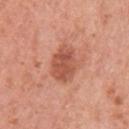Assessment:
Imaged during a routine full-body skin examination; the lesion was not biopsied and no histopathology is available.
Context:
The lesion's longest dimension is about 4 mm. The lesion is located on the arm. A close-up tile cropped from a whole-body skin photograph, about 15 mm across. The patient is a female approximately 50 years of age. The lesion-visualizer software estimated a footprint of about 11 mm², an outline eccentricity of about 0.65 (0 = round, 1 = elongated), and a symmetry-axis asymmetry near 0.15. And it measured a border-irregularity index near 1.5/10, a color-variation rating of about 3.5/10, and radial color variation of about 1. This is a white-light tile.